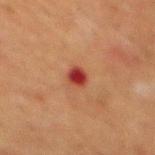No biopsy was performed on this lesion — it was imaged during a full skin examination and was not determined to be concerning.
A male patient, aged 48 to 52.
Located on the mid back.
Cropped from a total-body skin-imaging series; the visible field is about 15 mm.